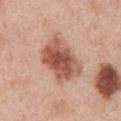This lesion was catalogued during total-body skin photography and was not selected for biopsy. The lesion is on the front of the torso. A male subject aged 68–72. Longest diameter approximately 6 mm. A roughly 15 mm field-of-view crop from a total-body skin photograph.